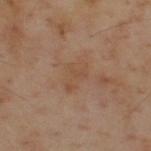This lesion was catalogued during total-body skin photography and was not selected for biopsy. Automated tile analysis of the lesion measured an outline eccentricity of about 0.8 (0 = round, 1 = elongated) and two-axis asymmetry of about 0.35. The analysis additionally found lesion-presence confidence of about 100/100. A close-up tile cropped from a whole-body skin photograph, about 15 mm across. The lesion is on the back. The tile uses cross-polarized illumination. A male patient in their mid- to late 50s. Longest diameter approximately 3.5 mm.